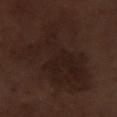| key | value |
|---|---|
| biopsy status | imaged on a skin check; not biopsied |
| illumination | white-light illumination |
| image | ~15 mm tile from a whole-body skin photo |
| lesion diameter | ≈10 mm |
| anatomic site | the right lower leg |
| patient | male, approximately 70 years of age |